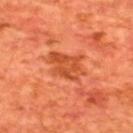follow-up: total-body-photography surveillance lesion; no biopsy
automated metrics: a mean CIELAB color near L≈47 a*≈33 b*≈40, a lesion–skin lightness drop of about 9, and a normalized border contrast of about 6.5; a border-irregularity rating of about 4/10, internal color variation of about 3 on a 0–10 scale, and peripheral color asymmetry of about 1
patient: male, aged approximately 65
imaging modality: ~15 mm crop, total-body skin-cancer survey
site: the back